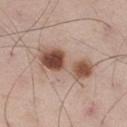Captured during whole-body skin photography for melanoma surveillance; the lesion was not biopsied. The lesion's longest dimension is about 7.5 mm. The tile uses white-light illumination. The subject is a male aged 53 to 57. A region of skin cropped from a whole-body photographic capture, roughly 15 mm wide. From the right thigh. The total-body-photography lesion software estimated an area of roughly 17 mm² and a shape eccentricity near 0.95. The software also gave an average lesion color of about L≈52 a*≈19 b*≈27 (CIELAB), roughly 15 lightness units darker than nearby skin, and a normalized border contrast of about 10. And it measured an automated nevus-likeness rating near 100 out of 100 and lesion-presence confidence of about 100/100.